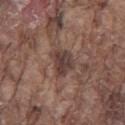Captured during whole-body skin photography for melanoma surveillance; the lesion was not biopsied. This is a white-light tile. The patient is a male aged 78–82. The lesion is located on the left thigh. A roughly 15 mm field-of-view crop from a total-body skin photograph. Automated tile analysis of the lesion measured a mean CIELAB color near L≈39 a*≈17 b*≈20 and a normalized border contrast of about 9. And it measured border irregularity of about 3 on a 0–10 scale, a color-variation rating of about 4.5/10, and a peripheral color-asymmetry measure near 1.5.